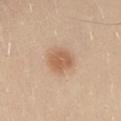follow-up: imaged on a skin check; not biopsied